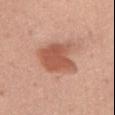biopsy_status: not biopsied; imaged during a skin examination
lesion_size:
  long_diameter_mm_approx: 5.5
image:
  source: total-body photography crop
  field_of_view_mm: 15
site: chest
patient:
  sex: female
  age_approx: 65
lighting: white-light